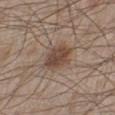| key | value |
|---|---|
| workup | imaged on a skin check; not biopsied |
| image | total-body-photography crop, ~15 mm field of view |
| subject | male, aged around 60 |
| body site | the left lower leg |